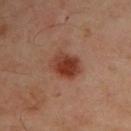notes: total-body-photography surveillance lesion; no biopsy
anatomic site: the left arm
subject: male, aged 48–52
imaging modality: 15 mm crop, total-body photography